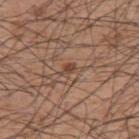This lesion was catalogued during total-body skin photography and was not selected for biopsy. The lesion is on the upper back. Measured at roughly 3 mm in maximum diameter. A male subject aged 58–62. A 15 mm close-up tile from a total-body photography series done for melanoma screening. The tile uses white-light illumination.